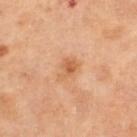Impression:
The lesion was photographed on a routine skin check and not biopsied; there is no pathology result.
Acquisition and patient details:
A female patient, aged 53–57. On the left thigh. A lesion tile, about 15 mm wide, cut from a 3D total-body photograph.